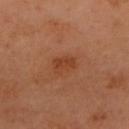biopsy status=imaged on a skin check; not biopsied | subject=female, about 60 years old | anatomic site=the head or neck | acquisition=~15 mm crop, total-body skin-cancer survey | size=~3 mm (longest diameter).